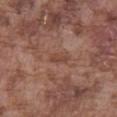No biopsy was performed on this lesion — it was imaged during a full skin examination and was not determined to be concerning. Automated tile analysis of the lesion measured a lesion color around L≈44 a*≈22 b*≈26 in CIELAB and about 7 CIELAB-L* units darker than the surrounding skin. And it measured a border-irregularity index near 3.5/10, a color-variation rating of about 0/10, and radial color variation of about 0. The subject is a male in their mid- to late 70s. A lesion tile, about 15 mm wide, cut from a 3D total-body photograph. Captured under white-light illumination. From the abdomen.